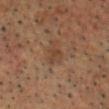* automated metrics — a lesion area of about 4.5 mm² and an eccentricity of roughly 0.8; border irregularity of about 5.5 on a 0–10 scale and peripheral color asymmetry of about 0.5
* lesion diameter — ≈3 mm
* imaging modality — ~15 mm crop, total-body skin-cancer survey
* patient — male, aged approximately 55
* illumination — cross-polarized
* anatomic site — the head or neck